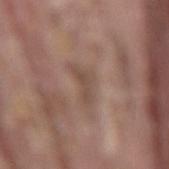biopsy status: catalogued during a skin exam; not biopsied
illumination: white-light illumination
body site: the back
diameter: ≈3.5 mm
automated lesion analysis: a footprint of about 5.5 mm² and a shape eccentricity near 0.75; an average lesion color of about L≈48 a*≈16 b*≈24 (CIELAB) and a lesion-to-skin contrast of about 5.5 (normalized; higher = more distinct); a color-variation rating of about 2/10; a classifier nevus-likeness of about 0/100 and lesion-presence confidence of about 75/100
patient: male, roughly 40 years of age
imaging modality: ~15 mm tile from a whole-body skin photo